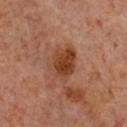Clinical impression: The lesion was tiled from a total-body skin photograph and was not biopsied. Background: Located on the front of the torso. Captured under cross-polarized illumination. The subject is a female aged around 60. This image is a 15 mm lesion crop taken from a total-body photograph.